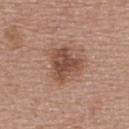Recorded during total-body skin imaging; not selected for excision or biopsy. The subject is a female roughly 60 years of age. Cropped from a whole-body photographic skin survey; the tile spans about 15 mm. The lesion is located on the upper back. Imaged with white-light lighting.